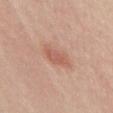subject=male, in their mid-60s | image-analysis metrics=a lesion area of about 5 mm² and a shape eccentricity near 0.85; a nevus-likeness score of about 80/100 and lesion-presence confidence of about 100/100 | image source=15 mm crop, total-body photography | anatomic site=the abdomen.